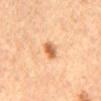Case summary:
– follow-up · imaged on a skin check; not biopsied
– lesion size · ≈3.5 mm
– patient · male, aged 83 or older
– acquisition · ~15 mm crop, total-body skin-cancer survey
– TBP lesion metrics · a shape eccentricity near 0.9 and two-axis asymmetry of about 0.25
– anatomic site · the mid back
– tile lighting · cross-polarized illumination